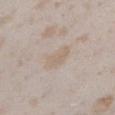| key | value |
|---|---|
| workup | no biopsy performed (imaged during a skin exam) |